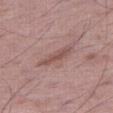– workup — total-body-photography surveillance lesion; no biopsy
– illumination — white-light
– automated metrics — a footprint of about 5 mm² and a shape eccentricity near 0.95; roughly 9 lightness units darker than nearby skin and a lesion-to-skin contrast of about 6.5 (normalized; higher = more distinct); a border-irregularity index near 3.5/10, internal color variation of about 1 on a 0–10 scale, and radial color variation of about 0.5
– site — the right thigh
– lesion size — ≈4.5 mm
– subject — male, aged 48–52
– acquisition — total-body-photography crop, ~15 mm field of view